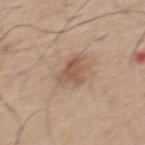notes: catalogued during a skin exam; not biopsied | image: 15 mm crop, total-body photography | location: the upper back | subject: male, in their mid- to late 60s | lesion size: ≈3 mm | lighting: white-light illumination.